Cropped from a whole-body photographic skin survey; the tile spans about 15 mm. A female subject, aged 58–62. On the mid back: 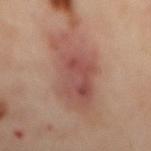Image and clinical context:
Approximately 10 mm at its widest.
Diagnosis:
On biopsy, histopathology showed a nodular basal cell carcinoma — a malignant lesion.